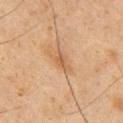| feature | finding |
|---|---|
| subject | male, in their 60s |
| acquisition | total-body-photography crop, ~15 mm field of view |
| site | the chest |